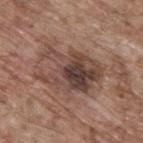The lesion was tiled from a total-body skin photograph and was not biopsied. Approximately 7 mm at its widest. An algorithmic analysis of the crop reported an outline eccentricity of about 0.75 (0 = round, 1 = elongated) and a shape-asymmetry score of about 0.35 (0 = symmetric). It also reported a mean CIELAB color near L≈43 a*≈17 b*≈23 and a normalized lesion–skin contrast near 9.5. The analysis additionally found border irregularity of about 7 on a 0–10 scale, a color-variation rating of about 8.5/10, and peripheral color asymmetry of about 3. The lesion is on the upper back. A close-up tile cropped from a whole-body skin photograph, about 15 mm across. The subject is a male in their 70s.